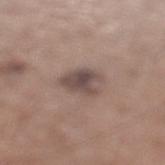Q: Was a biopsy performed?
A: no biopsy performed (imaged during a skin exam)
Q: Automated lesion metrics?
A: an area of roughly 9 mm², an outline eccentricity of about 0.7 (0 = round, 1 = elongated), and a shape-asymmetry score of about 0.25 (0 = symmetric); a border-irregularity rating of about 2.5/10, internal color variation of about 5.5 on a 0–10 scale, and peripheral color asymmetry of about 2
Q: How was this image acquired?
A: ~15 mm crop, total-body skin-cancer survey
Q: What are the patient's age and sex?
A: male, in their mid-60s
Q: Where on the body is the lesion?
A: the left lower leg
Q: Lesion size?
A: ≈4 mm
Q: Illumination type?
A: white-light